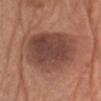{"biopsy_status": "not biopsied; imaged during a skin examination", "lighting": "white-light", "site": "left upper arm", "patient": {"sex": "female", "age_approx": 75}, "image": {"source": "total-body photography crop", "field_of_view_mm": 15}, "lesion_size": {"long_diameter_mm_approx": 7.5}}